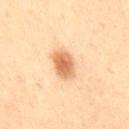<lesion>
  <biopsy_status>not biopsied; imaged during a skin examination</biopsy_status>
  <patient>
    <sex>male</sex>
    <age_approx>55</age_approx>
  </patient>
  <image>
    <source>total-body photography crop</source>
    <field_of_view_mm>15</field_of_view_mm>
  </image>
  <site>abdomen</site>
</lesion>